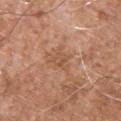Part of a total-body skin-imaging series; this lesion was reviewed on a skin check and was not flagged for biopsy. This is a white-light tile. A close-up tile cropped from a whole-body skin photograph, about 15 mm across. A male subject about 55 years old. Approximately 2.5 mm at its widest. From the upper back.